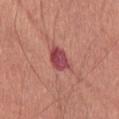{"biopsy_status": "not biopsied; imaged during a skin examination", "automated_metrics": {"vs_skin_contrast_norm": 10.0, "nevus_likeness_0_100": 0, "lesion_detection_confidence_0_100": 100}, "image": {"source": "total-body photography crop", "field_of_view_mm": 15}, "site": "right thigh", "lighting": "white-light", "patient": {"sex": "male", "age_approx": 65}, "lesion_size": {"long_diameter_mm_approx": 3.5}}